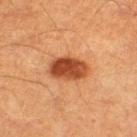follow-up: catalogued during a skin exam; not biopsied
TBP lesion metrics: an area of roughly 11 mm²; an automated nevus-likeness rating near 100 out of 100 and a lesion-detection confidence of about 100/100
image: ~15 mm crop, total-body skin-cancer survey
lighting: cross-polarized illumination
size: ~5 mm (longest diameter)
site: the right thigh
subject: male, aged around 60